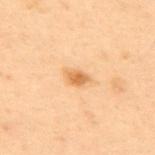The lesion was photographed on a routine skin check and not biopsied; there is no pathology result. This is a cross-polarized tile. An algorithmic analysis of the crop reported a lesion color around L≈55 a*≈19 b*≈37 in CIELAB, roughly 10 lightness units darker than nearby skin, and a normalized border contrast of about 7.5. The software also gave border irregularity of about 2 on a 0–10 scale and a peripheral color-asymmetry measure near 0.5. The subject is a male in their mid- to late 50s. The recorded lesion diameter is about 2.5 mm. From the upper back. A lesion tile, about 15 mm wide, cut from a 3D total-body photograph.